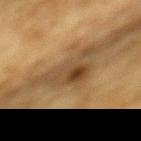{"biopsy_status": "not biopsied; imaged during a skin examination", "lesion_size": {"long_diameter_mm_approx": 6.0}, "patient": {"sex": "male", "age_approx": "85+"}, "lighting": "cross-polarized", "image": {"source": "total-body photography crop", "field_of_view_mm": 15}, "site": "mid back"}